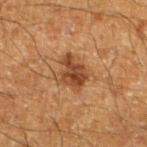Impression:
This lesion was catalogued during total-body skin photography and was not selected for biopsy.
Context:
On the left lower leg. This image is a 15 mm lesion crop taken from a total-body photograph. A male subject in their mid-60s. An algorithmic analysis of the crop reported border irregularity of about 3.5 on a 0–10 scale, internal color variation of about 4.5 on a 0–10 scale, and a peripheral color-asymmetry measure near 1.5.